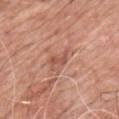– workup — imaged on a skin check; not biopsied
– imaging modality — 15 mm crop, total-body photography
– TBP lesion metrics — a shape eccentricity near 0.85; a border-irregularity index near 4.5/10, a color-variation rating of about 0/10, and peripheral color asymmetry of about 0
– size — ~2.5 mm (longest diameter)
– body site — the chest
– subject — male, approximately 60 years of age